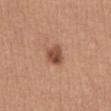{
  "biopsy_status": "not biopsied; imaged during a skin examination",
  "lesion_size": {
    "long_diameter_mm_approx": 2.5
  },
  "image": {
    "source": "total-body photography crop",
    "field_of_view_mm": 15
  },
  "site": "abdomen",
  "patient": {
    "sex": "female",
    "age_approx": 40
  },
  "lighting": "white-light"
}